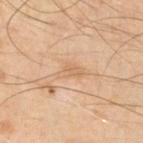{"biopsy_status": "not biopsied; imaged during a skin examination", "automated_metrics": {"cielab_L": 48, "cielab_a": 15, "cielab_b": 28, "vs_skin_darker_L": 5.0, "vs_skin_contrast_norm": 4.5}, "lesion_size": {"long_diameter_mm_approx": 2.5}, "site": "left upper arm", "patient": {"sex": "male", "age_approx": 50}, "image": {"source": "total-body photography crop", "field_of_view_mm": 15}}